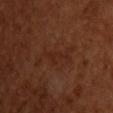The tile uses cross-polarized illumination.
A 15 mm close-up extracted from a 3D total-body photography capture.
Automated tile analysis of the lesion measured a footprint of about 3.5 mm², an eccentricity of roughly 0.9, and a shape-asymmetry score of about 0.6 (0 = symmetric). The analysis additionally found a border-irregularity index near 8/10. It also reported a lesion-detection confidence of about 100/100.
Located on the chest.
The subject is a female about 55 years old.
Approximately 4 mm at its widest.